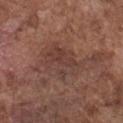Recorded during total-body skin imaging; not selected for excision or biopsy. On the chest. A 15 mm crop from a total-body photograph taken for skin-cancer surveillance. A male subject about 75 years old.